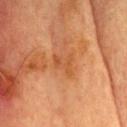Part of a total-body skin-imaging series; this lesion was reviewed on a skin check and was not flagged for biopsy. Cropped from a total-body skin-imaging series; the visible field is about 15 mm. A male subject, roughly 70 years of age. On the head or neck.